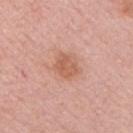Case summary:
- workup · catalogued during a skin exam; not biopsied
- image-analysis metrics · about 9 CIELAB-L* units darker than the surrounding skin and a lesion-to-skin contrast of about 6.5 (normalized; higher = more distinct); an automated nevus-likeness rating near 10 out of 100
- image source · total-body-photography crop, ~15 mm field of view
- lesion size · ~3.5 mm (longest diameter)
- location · the chest
- patient · male, aged around 65
- tile lighting · white-light